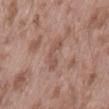Q: Was this lesion biopsied?
A: imaged on a skin check; not biopsied
Q: How was this image acquired?
A: ~15 mm tile from a whole-body skin photo
Q: Illumination type?
A: white-light
Q: Lesion size?
A: ≈3.5 mm
Q: Lesion location?
A: the lower back
Q: Automated lesion metrics?
A: a footprint of about 4.5 mm² and a shape-asymmetry score of about 0.45 (0 = symmetric); a border-irregularity rating of about 6/10, a within-lesion color-variation index near 1/10, and peripheral color asymmetry of about 0; a classifier nevus-likeness of about 0/100 and a lesion-detection confidence of about 95/100
Q: What are the patient's age and sex?
A: male, in their mid-50s